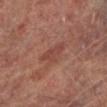{
  "lighting": "cross-polarized",
  "automated_metrics": {
    "area_mm2_approx": 6.5,
    "eccentricity": 0.75,
    "lesion_detection_confidence_0_100": 100
  },
  "patient": {
    "sex": "male",
    "age_approx": 65
  },
  "lesion_size": {
    "long_diameter_mm_approx": 3.5
  },
  "site": "leg",
  "image": {
    "source": "total-body photography crop",
    "field_of_view_mm": 15
  }
}